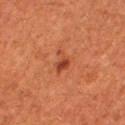Clinical impression:
Imaged during a routine full-body skin examination; the lesion was not biopsied and no histopathology is available.
Clinical summary:
The total-body-photography lesion software estimated border irregularity of about 6 on a 0–10 scale and radial color variation of about 0.5. The software also gave an automated nevus-likeness rating near 70 out of 100 and a lesion-detection confidence of about 100/100. Imaged with cross-polarized lighting. From the right thigh. This image is a 15 mm lesion crop taken from a total-body photograph. A female subject roughly 50 years of age.